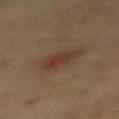Clinical impression: The lesion was photographed on a routine skin check and not biopsied; there is no pathology result. Context: The tile uses cross-polarized illumination. Automated image analysis of the tile measured a mean CIELAB color near L≈31 a*≈16 b*≈23, about 7 CIELAB-L* units darker than the surrounding skin, and a normalized lesion–skin contrast near 6.5. It also reported internal color variation of about 3 on a 0–10 scale and peripheral color asymmetry of about 1. A female patient aged around 55. On the mid back. The lesion's longest dimension is about 4.5 mm. A roughly 15 mm field-of-view crop from a total-body skin photograph.